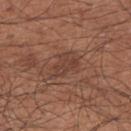biopsy status = no biopsy performed (imaged during a skin exam)
location = the right upper arm
image = ~15 mm crop, total-body skin-cancer survey
patient = male, in their mid-60s